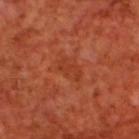workup — catalogued during a skin exam; not biopsied